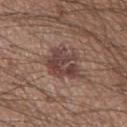Case summary:
- notes — imaged on a skin check; not biopsied
- location — the leg
- diameter — about 5.5 mm
- subject — male, approximately 20 years of age
- image source — 15 mm crop, total-body photography
- automated lesion analysis — a mean CIELAB color near L≈42 a*≈18 b*≈21, a lesion–skin lightness drop of about 10, and a lesion-to-skin contrast of about 8 (normalized; higher = more distinct); border irregularity of about 3.5 on a 0–10 scale, a within-lesion color-variation index near 5/10, and radial color variation of about 2
- lighting — white-light